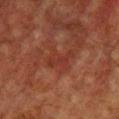Impression: Captured during whole-body skin photography for melanoma surveillance; the lesion was not biopsied. Image and clinical context: The lesion is on the front of the torso. A male subject about 60 years old. A 15 mm close-up extracted from a 3D total-body photography capture. Approximately 3.5 mm at its widest. This is a cross-polarized tile. Automated image analysis of the tile measured a footprint of about 4.5 mm², an outline eccentricity of about 0.9 (0 = round, 1 = elongated), and a symmetry-axis asymmetry near 0.5. The analysis additionally found a classifier nevus-likeness of about 0/100 and a detector confidence of about 95 out of 100 that the crop contains a lesion.